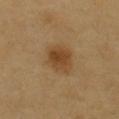| field | value |
|---|---|
| biopsy status | no biopsy performed (imaged during a skin exam) |
| acquisition | ~15 mm tile from a whole-body skin photo |
| patient | male, aged 58–62 |
| image-analysis metrics | a footprint of about 11 mm² and an eccentricity of roughly 0.55; an automated nevus-likeness rating near 95 out of 100 and a lesion-detection confidence of about 100/100 |
| anatomic site | the chest |
| size | about 4 mm |